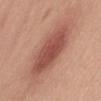Captured during whole-body skin photography for melanoma surveillance; the lesion was not biopsied. The tile uses white-light illumination. A region of skin cropped from a whole-body photographic capture, roughly 15 mm wide. A female patient aged around 50. Located on the abdomen.